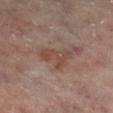notes = catalogued during a skin exam; not biopsied | subject = male, aged 63 to 67 | image = ~15 mm tile from a whole-body skin photo | site = the right lower leg.